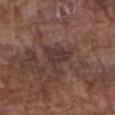– notes — total-body-photography surveillance lesion; no biopsy
– subject — male, aged approximately 75
– lighting — white-light
– acquisition — 15 mm crop, total-body photography
– anatomic site — the arm